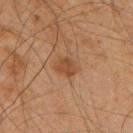* biopsy status: catalogued during a skin exam; not biopsied
* site: the mid back
* lesion size: about 3 mm
* acquisition: total-body-photography crop, ~15 mm field of view
* patient: male, approximately 65 years of age
* illumination: cross-polarized
* image-analysis metrics: an average lesion color of about L≈38 a*≈18 b*≈29 (CIELAB), a lesion–skin lightness drop of about 7, and a normalized lesion–skin contrast near 6.5; internal color variation of about 2 on a 0–10 scale and a peripheral color-asymmetry measure near 1